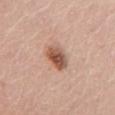{
  "biopsy_status": "not biopsied; imaged during a skin examination",
  "patient": {
    "sex": "female",
    "age_approx": 65
  },
  "lesion_size": {
    "long_diameter_mm_approx": 3.0
  },
  "site": "chest",
  "automated_metrics": {
    "eccentricity": 0.45,
    "border_irregularity_0_10": 2.0,
    "color_variation_0_10": 6.0,
    "peripheral_color_asymmetry": 2.0
  },
  "image": {
    "source": "total-body photography crop",
    "field_of_view_mm": 15
  }
}